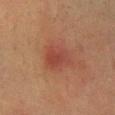Assessment: The lesion was tiled from a total-body skin photograph and was not biopsied. Image and clinical context: From the right lower leg. Cropped from a total-body skin-imaging series; the visible field is about 15 mm. A female patient, in their mid- to late 50s. This is a cross-polarized tile. About 4 mm across.